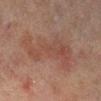A female patient aged 78 to 82.
A roughly 15 mm field-of-view crop from a total-body skin photograph.
The lesion is on the right lower leg.
Imaged with cross-polarized lighting.
Measured at roughly 9 mm in maximum diameter.